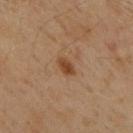Captured during whole-body skin photography for melanoma surveillance; the lesion was not biopsied. This image is a 15 mm lesion crop taken from a total-body photograph. Located on the upper back. The patient is a male in their 60s. The lesion's longest dimension is about 2.5 mm. The tile uses cross-polarized illumination.